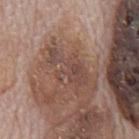Imaged during a routine full-body skin examination; the lesion was not biopsied and no histopathology is available. Measured at roughly 5.5 mm in maximum diameter. On the mid back. The subject is a male aged around 70. A lesion tile, about 15 mm wide, cut from a 3D total-body photograph. The tile uses white-light illumination.